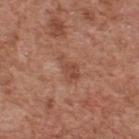Imaged during a routine full-body skin examination; the lesion was not biopsied and no histopathology is available. The lesion's longest dimension is about 3.5 mm. The lesion is on the upper back. The total-body-photography lesion software estimated a lesion area of about 5 mm², an eccentricity of roughly 0.85, and a symmetry-axis asymmetry near 0.4. It also reported a border-irregularity rating of about 4/10, a color-variation rating of about 2/10, and a peripheral color-asymmetry measure near 0.5. It also reported an automated nevus-likeness rating near 0 out of 100 and a detector confidence of about 100 out of 100 that the crop contains a lesion. Captured under white-light illumination. A male subject approximately 65 years of age. A close-up tile cropped from a whole-body skin photograph, about 15 mm across.